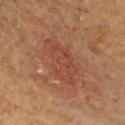This lesion was catalogued during total-body skin photography and was not selected for biopsy. Imaged with cross-polarized lighting. On the front of the torso. A 15 mm close-up extracted from a 3D total-body photography capture. The patient is a male aged 63–67.